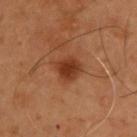workup = no biopsy performed (imaged during a skin exam); tile lighting = cross-polarized illumination; lesion diameter = about 3.5 mm; image source = 15 mm crop, total-body photography; site = the back; patient = male, approximately 55 years of age.